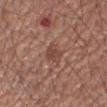A male patient aged 53–57. Longest diameter approximately 3 mm. This is a white-light tile. The lesion is located on the arm. A roughly 15 mm field-of-view crop from a total-body skin photograph. An algorithmic analysis of the crop reported a symmetry-axis asymmetry near 0.3. The software also gave a border-irregularity index near 2.5/10, a color-variation rating of about 2.5/10, and radial color variation of about 1.